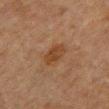biopsy status=imaged on a skin check; not biopsied
size=≈3.5 mm
anatomic site=the abdomen
imaging modality=total-body-photography crop, ~15 mm field of view
tile lighting=cross-polarized illumination
subject=male, about 80 years old